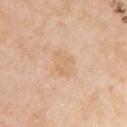patient=female, about 60 years old; imaging modality=15 mm crop, total-body photography; location=the left upper arm.